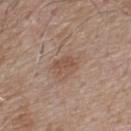Assessment: Imaged during a routine full-body skin examination; the lesion was not biopsied and no histopathology is available. Background: Longest diameter approximately 3 mm. The tile uses white-light illumination. From the upper back. Automated tile analysis of the lesion measured an area of roughly 5 mm², an eccentricity of roughly 0.75, and two-axis asymmetry of about 0.3. The analysis additionally found an average lesion color of about L≈51 a*≈18 b*≈27 (CIELAB) and about 7 CIELAB-L* units darker than the surrounding skin. The software also gave border irregularity of about 3 on a 0–10 scale and a peripheral color-asymmetry measure near 0.5. The analysis additionally found a nevus-likeness score of about 5/100. The subject is a male aged around 55. A roughly 15 mm field-of-view crop from a total-body skin photograph.